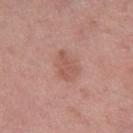Q: Lesion location?
A: the right thigh
Q: Patient demographics?
A: male, aged 53–57
Q: How large is the lesion?
A: about 3.5 mm
Q: How was this image acquired?
A: ~15 mm tile from a whole-body skin photo
Q: How was the tile lit?
A: white-light illumination
Q: What did automated image analysis measure?
A: a footprint of about 7.5 mm², an outline eccentricity of about 0.7 (0 = round, 1 = elongated), and a shape-asymmetry score of about 0.25 (0 = symmetric); border irregularity of about 2.5 on a 0–10 scale, a within-lesion color-variation index near 1.5/10, and radial color variation of about 0.5; a classifier nevus-likeness of about 5/100 and a detector confidence of about 100 out of 100 that the crop contains a lesion